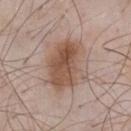Notes:
- follow-up: imaged on a skin check; not biopsied
- diameter: ~6.5 mm (longest diameter)
- location: the chest
- image-analysis metrics: a border-irregularity index near 3/10 and a peripheral color-asymmetry measure near 2.5
- tile lighting: white-light illumination
- patient: male, about 55 years old
- imaging modality: ~15 mm tile from a whole-body skin photo